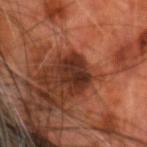Assessment: The lesion was photographed on a routine skin check and not biopsied; there is no pathology result. Context: A male subject, aged 68–72. From the head or neck. The total-body-photography lesion software estimated a lesion area of about 9 mm². It also reported about 12 CIELAB-L* units darker than the surrounding skin and a lesion-to-skin contrast of about 12 (normalized; higher = more distinct). And it measured a border-irregularity rating of about 2.5/10, a color-variation rating of about 4.5/10, and radial color variation of about 1.5. The software also gave a classifier nevus-likeness of about 10/100 and lesion-presence confidence of about 100/100. Imaged with cross-polarized lighting. The recorded lesion diameter is about 3.5 mm. This image is a 15 mm lesion crop taken from a total-body photograph.